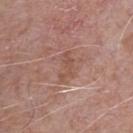The lesion was tiled from a total-body skin photograph and was not biopsied.
Imaged with white-light lighting.
On the chest.
The recorded lesion diameter is about 3.5 mm.
Cropped from a total-body skin-imaging series; the visible field is about 15 mm.
A male patient roughly 65 years of age.